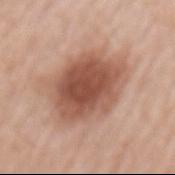Clinical impression:
Captured during whole-body skin photography for melanoma surveillance; the lesion was not biopsied.
Context:
The lesion's longest dimension is about 7 mm. A lesion tile, about 15 mm wide, cut from a 3D total-body photograph. Located on the mid back. A female patient, aged 68 to 72. The tile uses white-light illumination. The total-body-photography lesion software estimated a color-variation rating of about 5/10 and a peripheral color-asymmetry measure near 1.5.